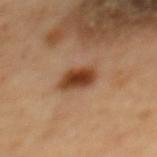workup: catalogued during a skin exam; not biopsied | illumination: cross-polarized illumination | TBP lesion metrics: an outline eccentricity of about 0.75 (0 = round, 1 = elongated); a lesion color around L≈42 a*≈23 b*≈34 in CIELAB and a lesion–skin lightness drop of about 15; border irregularity of about 2 on a 0–10 scale, a color-variation rating of about 5/10, and a peripheral color-asymmetry measure near 1.5; a nevus-likeness score of about 100/100 and a detector confidence of about 100 out of 100 that the crop contains a lesion | location: the mid back | patient: male, roughly 65 years of age | acquisition: ~15 mm crop, total-body skin-cancer survey | lesion diameter: ≈3.5 mm.